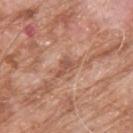notes = catalogued during a skin exam; not biopsied | image = 15 mm crop, total-body photography | patient = male, roughly 80 years of age | location = the upper back.